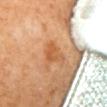The lesion was tiled from a total-body skin photograph and was not biopsied.
The lesion is on the upper back.
A 15 mm close-up tile from a total-body photography series done for melanoma screening.
Automated tile analysis of the lesion measured an average lesion color of about L≈59 a*≈26 b*≈42 (CIELAB), a lesion–skin lightness drop of about 9, and a lesion-to-skin contrast of about 6.5 (normalized; higher = more distinct). It also reported internal color variation of about 2.5 on a 0–10 scale and radial color variation of about 0.5. And it measured a detector confidence of about 100 out of 100 that the crop contains a lesion.
A female patient.
The tile uses cross-polarized illumination.
Longest diameter approximately 3.5 mm.